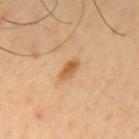<case>
<biopsy_status>not biopsied; imaged during a skin examination</biopsy_status>
<image>
  <source>total-body photography crop</source>
  <field_of_view_mm>15</field_of_view_mm>
</image>
<lesion_size>
  <long_diameter_mm_approx>3.0</long_diameter_mm_approx>
</lesion_size>
<patient>
  <sex>male</sex>
  <age_approx>65</age_approx>
</patient>
<lighting>cross-polarized</lighting>
<site>mid back</site>
</case>